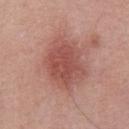Findings:
– biopsy status — total-body-photography surveillance lesion; no biopsy
– illumination — white-light
– lesion size — ~6.5 mm (longest diameter)
– anatomic site — the front of the torso
– patient — male, aged 53–57
– imaging modality — total-body-photography crop, ~15 mm field of view